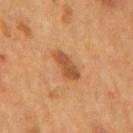The lesion was tiled from a total-body skin photograph and was not biopsied. The lesion-visualizer software estimated an area of roughly 7 mm², an eccentricity of roughly 0.9, and a symmetry-axis asymmetry near 0.2. The analysis additionally found border irregularity of about 2.5 on a 0–10 scale, a color-variation rating of about 2/10, and peripheral color asymmetry of about 0.5. It also reported an automated nevus-likeness rating near 90 out of 100 and lesion-presence confidence of about 100/100. A roughly 15 mm field-of-view crop from a total-body skin photograph. A male subject aged 53 to 57. The lesion is located on the mid back. Captured under cross-polarized illumination.